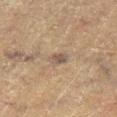Imaged during a routine full-body skin examination; the lesion was not biopsied and no histopathology is available. Automated image analysis of the tile measured a lesion color around L≈42 a*≈11 b*≈21 in CIELAB and a normalized lesion–skin contrast near 7.5. The analysis additionally found border irregularity of about 1.5 on a 0–10 scale, a within-lesion color-variation index near 2.5/10, and radial color variation of about 0.5. The lesion is located on the leg. The lesion's longest dimension is about 2.5 mm. Captured under cross-polarized illumination. A 15 mm close-up extracted from a 3D total-body photography capture. A male patient approximately 75 years of age.